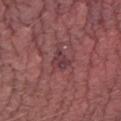Recorded during total-body skin imaging; not selected for excision or biopsy. The lesion is on the right thigh. The subject is a male aged around 50. A 15 mm close-up tile from a total-body photography series done for melanoma screening. The lesion's longest dimension is about 2.5 mm.